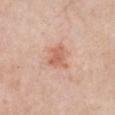An algorithmic analysis of the crop reported an area of roughly 8.5 mm² and a shape eccentricity near 0.4.
Located on the chest.
A close-up tile cropped from a whole-body skin photograph, about 15 mm across.
About 3.5 mm across.
A female subject aged approximately 60.